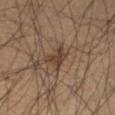No biopsy was performed on this lesion — it was imaged during a full skin examination and was not determined to be concerning.
Captured under cross-polarized illumination.
A male patient approximately 40 years of age.
The total-body-photography lesion software estimated two-axis asymmetry of about 0.45. The analysis additionally found a nevus-likeness score of about 65/100 and a detector confidence of about 95 out of 100 that the crop contains a lesion.
The lesion is located on the leg.
Measured at roughly 3.5 mm in maximum diameter.
A close-up tile cropped from a whole-body skin photograph, about 15 mm across.